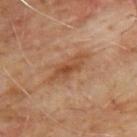notes: no biopsy performed (imaged during a skin exam)
automated metrics: a lesion color around L≈46 a*≈22 b*≈33 in CIELAB and a normalized lesion–skin contrast near 7.5
site: the upper back
subject: male, in their mid-60s
lesion diameter: ~5 mm (longest diameter)
illumination: cross-polarized illumination
acquisition: ~15 mm tile from a whole-body skin photo